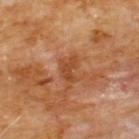Q: Is there a histopathology result?
A: catalogued during a skin exam; not biopsied
Q: Patient demographics?
A: male, in their 60s
Q: How large is the lesion?
A: ≈3 mm
Q: What is the anatomic site?
A: the chest
Q: What kind of image is this?
A: ~15 mm crop, total-body skin-cancer survey
Q: Illumination type?
A: cross-polarized illumination